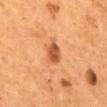Longest diameter approximately 2.5 mm. On the mid back. This is a cross-polarized tile. The subject is a male approximately 55 years of age. A roughly 15 mm field-of-view crop from a total-body skin photograph.